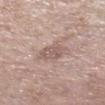Case summary:
* lesion size — ≈2.5 mm
* subject — female, in their mid- to late 80s or older
* location — the right lower leg
* image source — total-body-photography crop, ~15 mm field of view